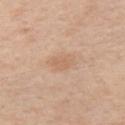Assessment: Recorded during total-body skin imaging; not selected for excision or biopsy. Clinical summary: The subject is a male approximately 60 years of age. A roughly 15 mm field-of-view crop from a total-body skin photograph. Measured at roughly 3.5 mm in maximum diameter. Located on the left upper arm.